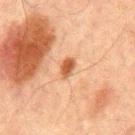Case summary:
– workup: catalogued during a skin exam; not biopsied